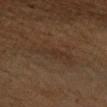The lesion was photographed on a routine skin check and not biopsied; there is no pathology result. A male patient, aged 58–62. A lesion tile, about 15 mm wide, cut from a 3D total-body photograph. From the left forearm. Imaged with cross-polarized lighting. Measured at roughly 5.5 mm in maximum diameter.